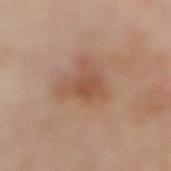A female patient, roughly 50 years of age. A 15 mm close-up tile from a total-body photography series done for melanoma screening. Located on the abdomen.